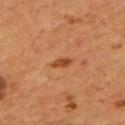No biopsy was performed on this lesion — it was imaged during a full skin examination and was not determined to be concerning. The lesion is on the mid back. A roughly 15 mm field-of-view crop from a total-body skin photograph. The recorded lesion diameter is about 2.5 mm. The tile uses cross-polarized illumination. The patient is a male in their mid- to late 50s.